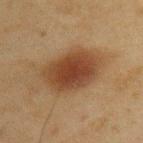notes: catalogued during a skin exam; not biopsied | lesion size: about 7 mm | anatomic site: the left upper arm | TBP lesion metrics: a nevus-likeness score of about 100/100 | acquisition: total-body-photography crop, ~15 mm field of view | subject: male, aged 43 to 47.